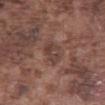{
  "biopsy_status": "not biopsied; imaged during a skin examination",
  "image": {
    "source": "total-body photography crop",
    "field_of_view_mm": 15
  },
  "patient": {
    "sex": "male",
    "age_approx": 75
  },
  "site": "abdomen",
  "automated_metrics": {
    "area_mm2_approx": 7.0,
    "eccentricity": 0.8,
    "shape_asymmetry": 0.2,
    "cielab_L": 41,
    "cielab_a": 18,
    "cielab_b": 22,
    "vs_skin_darker_L": 6.0,
    "vs_skin_contrast_norm": 5.5,
    "border_irregularity_0_10": 2.0,
    "color_variation_0_10": 4.0,
    "peripheral_color_asymmetry": 1.5,
    "nevus_likeness_0_100": 0,
    "lesion_detection_confidence_0_100": 70
  }
}